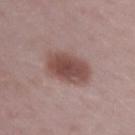Context: A female subject, roughly 45 years of age. A roughly 15 mm field-of-view crop from a total-body skin photograph. Approximately 5 mm at its widest. On the right upper arm. Imaged with white-light lighting. The total-body-photography lesion software estimated a symmetry-axis asymmetry near 0.15. The software also gave a mean CIELAB color near L≈48 a*≈20 b*≈22 and a lesion-to-skin contrast of about 9 (normalized; higher = more distinct). The analysis additionally found a border-irregularity index near 1.5/10, a within-lesion color-variation index near 3.5/10, and a peripheral color-asymmetry measure near 1. The software also gave a lesion-detection confidence of about 100/100.